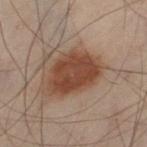Case summary:
- acquisition · ~15 mm tile from a whole-body skin photo
- location · the left thigh
- patient · male, aged around 50